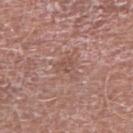This lesion was catalogued during total-body skin photography and was not selected for biopsy.
Imaged with white-light lighting.
Cropped from a total-body skin-imaging series; the visible field is about 15 mm.
A male patient about 70 years old.
The lesion is located on the right upper arm.
Approximately 2.5 mm at its widest.
The total-body-photography lesion software estimated an area of roughly 4.5 mm², an outline eccentricity of about 0.6 (0 = round, 1 = elongated), and a symmetry-axis asymmetry near 0.55. It also reported an average lesion color of about L≈52 a*≈21 b*≈24 (CIELAB) and a normalized border contrast of about 4.5.